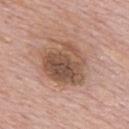biopsy status: catalogued during a skin exam; not biopsied
acquisition: total-body-photography crop, ~15 mm field of view
lesion diameter: ≈6 mm
anatomic site: the mid back
image-analysis metrics: an area of roughly 22 mm² and a symmetry-axis asymmetry near 0.2; a lesion color around L≈52 a*≈19 b*≈28 in CIELAB and a lesion-to-skin contrast of about 8.5 (normalized; higher = more distinct)
subject: male, in their mid- to late 70s
tile lighting: white-light illumination